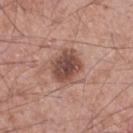Notes:
– biopsy status — total-body-photography surveillance lesion; no biopsy
– anatomic site — the right thigh
– lesion diameter — ≈4 mm
– subject — male, aged approximately 55
– tile lighting — white-light illumination
– imaging modality — total-body-photography crop, ~15 mm field of view